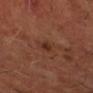Notes:
– notes · imaged on a skin check; not biopsied
– patient · male, aged around 60
– acquisition · 15 mm crop, total-body photography
– automated metrics · an average lesion color of about L≈28 a*≈21 b*≈26 (CIELAB), about 7 CIELAB-L* units darker than the surrounding skin, and a normalized lesion–skin contrast near 7; border irregularity of about 2 on a 0–10 scale, internal color variation of about 1 on a 0–10 scale, and radial color variation of about 0.5; a classifier nevus-likeness of about 25/100 and lesion-presence confidence of about 100/100
– anatomic site · the left forearm
– size · ≈1.5 mm